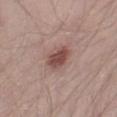The lesion was tiled from a total-body skin photograph and was not biopsied. Approximately 3.5 mm at its widest. The tile uses white-light illumination. On the right thigh. A male patient about 40 years old. A 15 mm close-up extracted from a 3D total-body photography capture.